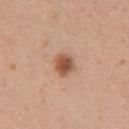Imaged during a routine full-body skin examination; the lesion was not biopsied and no histopathology is available.
Captured under white-light illumination.
A female subject approximately 30 years of age.
The lesion's longest dimension is about 3 mm.
From the chest.
A close-up tile cropped from a whole-body skin photograph, about 15 mm across.
Automated tile analysis of the lesion measured an average lesion color of about L≈54 a*≈22 b*≈32 (CIELAB). The analysis additionally found a lesion-detection confidence of about 100/100.